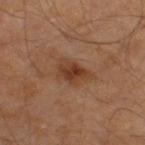This lesion was catalogued during total-body skin photography and was not selected for biopsy. Measured at roughly 4 mm in maximum diameter. On the right thigh. The subject is a male roughly 60 years of age. Automated image analysis of the tile measured an area of roughly 7 mm², a shape eccentricity near 0.8, and a shape-asymmetry score of about 0.35 (0 = symmetric). The software also gave border irregularity of about 3.5 on a 0–10 scale, a color-variation rating of about 4/10, and peripheral color asymmetry of about 1. A lesion tile, about 15 mm wide, cut from a 3D total-body photograph.